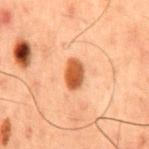Assessment: No biopsy was performed on this lesion — it was imaged during a full skin examination and was not determined to be concerning. Background: A roughly 15 mm field-of-view crop from a total-body skin photograph. A male subject, roughly 50 years of age. Imaged with cross-polarized lighting. Measured at roughly 3.5 mm in maximum diameter. The lesion is located on the chest.